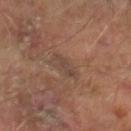This lesion was catalogued during total-body skin photography and was not selected for biopsy.
A male patient roughly 65 years of age.
A roughly 15 mm field-of-view crop from a total-body skin photograph.
The lesion is located on the right lower leg.